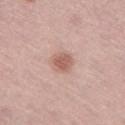Recorded during total-body skin imaging; not selected for excision or biopsy. The subject is a female aged 58 to 62. Cropped from a whole-body photographic skin survey; the tile spans about 15 mm. The lesion is on the left thigh.